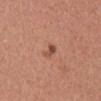follow-up: no biopsy performed (imaged during a skin exam)
lesion diameter: ~2.5 mm (longest diameter)
image: ~15 mm tile from a whole-body skin photo
patient: male, approximately 45 years of age
tile lighting: white-light illumination
anatomic site: the mid back